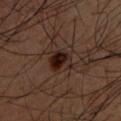{
  "site": "back",
  "patient": {
    "sex": "male",
    "age_approx": 50
  },
  "image": {
    "source": "total-body photography crop",
    "field_of_view_mm": 15
  }
}